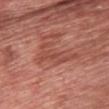Findings:
* biopsy status: catalogued during a skin exam; not biopsied
* image source: total-body-photography crop, ~15 mm field of view
* body site: the front of the torso
* subject: male, aged 58–62